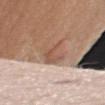The lesion was tiled from a total-body skin photograph and was not biopsied. A 15 mm close-up extracted from a 3D total-body photography capture. The lesion is located on the arm. Imaged with white-light lighting. A male subject, aged 73 to 77.